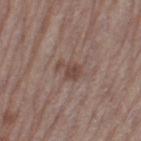Recorded during total-body skin imaging; not selected for excision or biopsy.
Cropped from a whole-body photographic skin survey; the tile spans about 15 mm.
The patient is a female aged around 65.
On the left thigh.